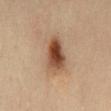  biopsy_status: not biopsied; imaged during a skin examination
  patient:
    sex: female
    age_approx: 40
  lesion_size:
    long_diameter_mm_approx: 5.0
  site: abdomen
  lighting: cross-polarized
  image:
    source: total-body photography crop
    field_of_view_mm: 15